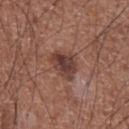Assessment: The lesion was photographed on a routine skin check and not biopsied; there is no pathology result. Context: The total-body-photography lesion software estimated an area of roughly 8.5 mm² and two-axis asymmetry of about 0.3. The software also gave a mean CIELAB color near L≈40 a*≈21 b*≈23 and roughly 11 lightness units darker than nearby skin. The lesion's longest dimension is about 4 mm. A male subject in their mid- to late 70s. A 15 mm close-up tile from a total-body photography series done for melanoma screening. Located on the chest.